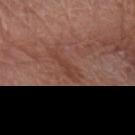Impression:
Captured during whole-body skin photography for melanoma surveillance; the lesion was not biopsied.
Image and clinical context:
About 4.5 mm across. A female subject aged 78–82. The lesion is located on the arm. Cropped from a total-body skin-imaging series; the visible field is about 15 mm. The lesion-visualizer software estimated a lesion area of about 5 mm², an eccentricity of roughly 0.95, and a shape-asymmetry score of about 0.45 (0 = symmetric).